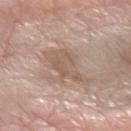Captured during whole-body skin photography for melanoma surveillance; the lesion was not biopsied.
A lesion tile, about 15 mm wide, cut from a 3D total-body photograph.
The recorded lesion diameter is about 5 mm.
From the left forearm.
A female subject, aged 63–67.
The lesion-visualizer software estimated a mean CIELAB color near L≈57 a*≈16 b*≈26, a lesion–skin lightness drop of about 8, and a lesion-to-skin contrast of about 5.5 (normalized; higher = more distinct). It also reported border irregularity of about 5 on a 0–10 scale, internal color variation of about 3 on a 0–10 scale, and a peripheral color-asymmetry measure near 1. The software also gave lesion-presence confidence of about 95/100.
The tile uses white-light illumination.